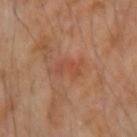<case>
<patient>
  <sex>female</sex>
  <age_approx>45</age_approx>
</patient>
<image>
  <source>total-body photography crop</source>
  <field_of_view_mm>15</field_of_view_mm>
</image>
<lighting>cross-polarized</lighting>
<automated_metrics>
  <cielab_L>48</cielab_L>
  <cielab_a>26</cielab_a>
  <cielab_b>32</cielab_b>
  <vs_skin_darker_L>6.0</vs_skin_darker_L>
  <border_irregularity_0_10>6.0</border_irregularity_0_10>
  <peripheral_color_asymmetry>0.0</peripheral_color_asymmetry>
  <lesion_detection_confidence_0_100>100</lesion_detection_confidence_0_100>
</automated_metrics>
<lesion_size>
  <long_diameter_mm_approx>3.5</long_diameter_mm_approx>
</lesion_size>
<site>right forearm</site>
</case>